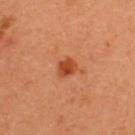{
  "biopsy_status": "not biopsied; imaged during a skin examination",
  "image": {
    "source": "total-body photography crop",
    "field_of_view_mm": 15
  },
  "lesion_size": {
    "long_diameter_mm_approx": 2.5
  },
  "site": "back",
  "patient": {
    "sex": "male",
    "age_approx": 35
  }
}